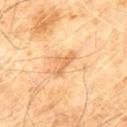This lesion was catalogued during total-body skin photography and was not selected for biopsy. A male patient, aged approximately 60. An algorithmic analysis of the crop reported an area of roughly 4 mm², an eccentricity of roughly 0.9, and two-axis asymmetry of about 0.6. The software also gave a lesion color around L≈67 a*≈22 b*≈43 in CIELAB and about 9 CIELAB-L* units darker than the surrounding skin. And it measured a border-irregularity index near 6/10, a color-variation rating of about 0.5/10, and a peripheral color-asymmetry measure near 0. About 3.5 mm across. Captured under cross-polarized illumination. Located on the chest. A lesion tile, about 15 mm wide, cut from a 3D total-body photograph.